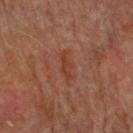| field | value |
|---|---|
| notes | imaged on a skin check; not biopsied |
| illumination | cross-polarized |
| subject | male, aged 73 to 77 |
| imaging modality | total-body-photography crop, ~15 mm field of view |
| lesion diameter | about 3.5 mm |
| anatomic site | the right upper arm |